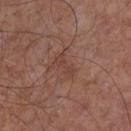Clinical impression:
Captured during whole-body skin photography for melanoma surveillance; the lesion was not biopsied.
Context:
Located on the chest. A region of skin cropped from a whole-body photographic capture, roughly 15 mm wide. A male subject, in their mid- to late 50s. Automated tile analysis of the lesion measured a lesion area of about 3.5 mm², a shape eccentricity near 0.9, and a shape-asymmetry score of about 0.45 (0 = symmetric). The lesion's longest dimension is about 3 mm. This is a white-light tile.